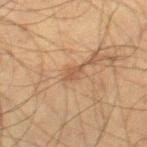workup = no biopsy performed (imaged during a skin exam) | subject = male, aged 58 to 62 | automated lesion analysis = a mean CIELAB color near L≈45 a*≈17 b*≈29, a lesion–skin lightness drop of about 7, and a normalized border contrast of about 6 | acquisition = ~15 mm crop, total-body skin-cancer survey | lesion size = ≈3 mm | lighting = cross-polarized | anatomic site = the right thigh.